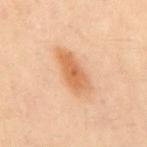{
  "biopsy_status": "not biopsied; imaged during a skin examination",
  "patient": {
    "sex": "male",
    "age_approx": 50
  },
  "lesion_size": {
    "long_diameter_mm_approx": 5.5
  },
  "site": "mid back",
  "automated_metrics": {
    "shape_asymmetry": 0.25,
    "border_irregularity_0_10": 3.0,
    "color_variation_0_10": 3.5,
    "peripheral_color_asymmetry": 1.0,
    "nevus_likeness_0_100": 100,
    "lesion_detection_confidence_0_100": 100
  },
  "image": {
    "source": "total-body photography crop",
    "field_of_view_mm": 15
  }
}